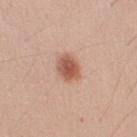– notes: imaged on a skin check; not biopsied
– subject: male, aged approximately 30
– body site: the right upper arm
– image: ~15 mm tile from a whole-body skin photo